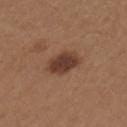The lesion's longest dimension is about 3.5 mm. A 15 mm close-up extracted from a 3D total-body photography capture. A female patient, in their 40s. Captured under white-light illumination. An algorithmic analysis of the crop reported a lesion area of about 8 mm². It also reported a lesion color around L≈38 a*≈20 b*≈27 in CIELAB, a lesion–skin lightness drop of about 12, and a lesion-to-skin contrast of about 10 (normalized; higher = more distinct). It also reported border irregularity of about 2 on a 0–10 scale and a peripheral color-asymmetry measure near 0.5. It also reported a nevus-likeness score of about 90/100 and a detector confidence of about 100 out of 100 that the crop contains a lesion. On the right upper arm.